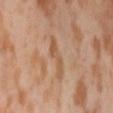notes: catalogued during a skin exam; not biopsied